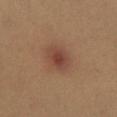Findings:
• workup · total-body-photography surveillance lesion; no biopsy
• body site · the left thigh
• tile lighting · cross-polarized
• diameter · ≈4 mm
• subject · female, aged 28–32
• acquisition · ~15 mm tile from a whole-body skin photo